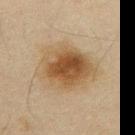Imaged during a routine full-body skin examination; the lesion was not biopsied and no histopathology is available.